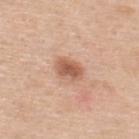A lesion tile, about 15 mm wide, cut from a 3D total-body photograph.
On the upper back.
A male patient, aged approximately 50.
Approximately 3 mm at its widest.